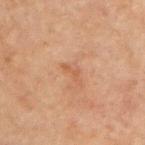notes: total-body-photography surveillance lesion; no biopsy | size: ~2.5 mm (longest diameter) | anatomic site: the chest | image: ~15 mm tile from a whole-body skin photo | subject: male, aged approximately 70.